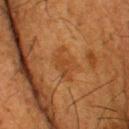follow-up: total-body-photography surveillance lesion; no biopsy
body site: the head or neck
tile lighting: cross-polarized
acquisition: ~15 mm tile from a whole-body skin photo
lesion size: ≈4 mm
subject: male, in their mid-60s
automated lesion analysis: a lesion area of about 7 mm² and two-axis asymmetry of about 0.4; a mean CIELAB color near L≈36 a*≈21 b*≈34, roughly 5 lightness units darker than nearby skin, and a normalized border contrast of about 4.5; a border-irregularity index near 5/10 and a color-variation rating of about 2/10